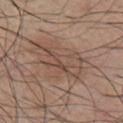Imaged with white-light lighting. A 15 mm crop from a total-body photograph taken for skin-cancer surveillance. The lesion's longest dimension is about 6.5 mm. Located on the chest. Automated image analysis of the tile measured a lesion area of about 14 mm², an outline eccentricity of about 0.9 (0 = round, 1 = elongated), and two-axis asymmetry of about 0.55. A male subject, in their mid- to late 50s.